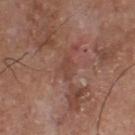This lesion was catalogued during total-body skin photography and was not selected for biopsy.
Located on the chest.
Automated image analysis of the tile measured a lesion area of about 3.5 mm², a shape eccentricity near 0.85, and two-axis asymmetry of about 0.45. The analysis additionally found an automated nevus-likeness rating near 0 out of 100 and a detector confidence of about 55 out of 100 that the crop contains a lesion.
The lesion's longest dimension is about 3 mm.
Cropped from a total-body skin-imaging series; the visible field is about 15 mm.
The tile uses white-light illumination.
A male patient, aged around 75.